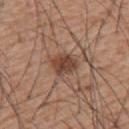| feature | finding |
|---|---|
| workup | catalogued during a skin exam; not biopsied |
| diameter | ~3.5 mm (longest diameter) |
| imaging modality | 15 mm crop, total-body photography |
| image-analysis metrics | a footprint of about 7 mm², an outline eccentricity of about 0.6 (0 = round, 1 = elongated), and two-axis asymmetry of about 0.2; a border-irregularity index near 2/10, internal color variation of about 3.5 on a 0–10 scale, and radial color variation of about 1; an automated nevus-likeness rating near 90 out of 100 and a detector confidence of about 100 out of 100 that the crop contains a lesion |
| subject | male, aged approximately 55 |
| anatomic site | the upper back |
| illumination | white-light |